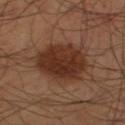This lesion was catalogued during total-body skin photography and was not selected for biopsy.
A male subject, aged 53 to 57.
A region of skin cropped from a whole-body photographic capture, roughly 15 mm wide.
This is a cross-polarized tile.
From the leg.
Automated tile analysis of the lesion measured a lesion area of about 23 mm², an eccentricity of roughly 0.5, and a symmetry-axis asymmetry near 0.15. And it measured an average lesion color of about L≈31 a*≈21 b*≈27 (CIELAB), roughly 11 lightness units darker than nearby skin, and a lesion-to-skin contrast of about 10.5 (normalized; higher = more distinct). And it measured a peripheral color-asymmetry measure near 1.5. The software also gave a classifier nevus-likeness of about 100/100 and a lesion-detection confidence of about 100/100.